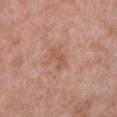<lesion>
<biopsy_status>not biopsied; imaged during a skin examination</biopsy_status>
<image>
  <source>total-body photography crop</source>
  <field_of_view_mm>15</field_of_view_mm>
</image>
<lighting>white-light</lighting>
<patient>
  <sex>female</sex>
  <age_approx>60</age_approx>
</patient>
<site>chest</site>
</lesion>